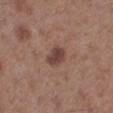| key | value |
|---|---|
| biopsy status | imaged on a skin check; not biopsied |
| patient | male, aged 58–62 |
| diameter | about 3 mm |
| automated metrics | a lesion area of about 5 mm² and an eccentricity of roughly 0.6; a lesion-detection confidence of about 100/100 |
| lighting | white-light illumination |
| anatomic site | the left lower leg |
| imaging modality | ~15 mm tile from a whole-body skin photo |